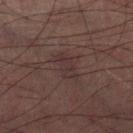Findings:
* biopsy status — catalogued during a skin exam; not biopsied
* patient — male, aged 58–62
* acquisition — 15 mm crop, total-body photography